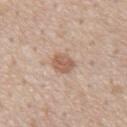Captured during whole-body skin photography for melanoma surveillance; the lesion was not biopsied. This is a white-light tile. The lesion is on the abdomen. A male subject aged approximately 55. Cropped from a whole-body photographic skin survey; the tile spans about 15 mm.